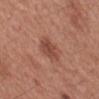Findings:
- workup — no biopsy performed (imaged during a skin exam)
- image-analysis metrics — an area of roughly 7.5 mm², a shape eccentricity near 0.7, and two-axis asymmetry of about 0.15; a border-irregularity rating of about 2/10 and a within-lesion color-variation index near 2.5/10
- lighting — white-light
- acquisition — 15 mm crop, total-body photography
- anatomic site — the left forearm
- patient — female, aged 48 to 52
- lesion diameter — about 3.5 mm